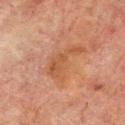Q: Was a biopsy performed?
A: imaged on a skin check; not biopsied
Q: How was the tile lit?
A: cross-polarized illumination
Q: Lesion location?
A: the upper back
Q: Who is the patient?
A: male, aged approximately 65
Q: Automated lesion metrics?
A: a border-irregularity index near 7.5/10 and radial color variation of about 1
Q: What is the imaging modality?
A: 15 mm crop, total-body photography